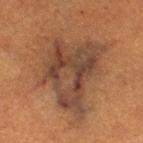Imaged during a routine full-body skin examination; the lesion was not biopsied and no histopathology is available.
The patient is a female aged around 55.
About 9 mm across.
On the right thigh.
A 15 mm close-up tile from a total-body photography series done for melanoma screening.